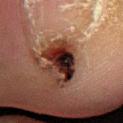{"biopsy_status": "not biopsied; imaged during a skin examination"}